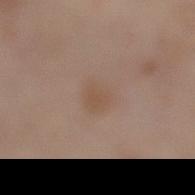Assessment:
Recorded during total-body skin imaging; not selected for excision or biopsy.
Acquisition and patient details:
The tile uses white-light illumination. The patient is a female in their mid-50s. The lesion is on the left lower leg. Cropped from a total-body skin-imaging series; the visible field is about 15 mm. An algorithmic analysis of the crop reported an area of roughly 5 mm², an outline eccentricity of about 0.5 (0 = round, 1 = elongated), and a shape-asymmetry score of about 0.25 (0 = symmetric). It also reported a mean CIELAB color near L≈51 a*≈15 b*≈28, a lesion–skin lightness drop of about 5, and a normalized lesion–skin contrast near 5.